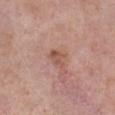{
  "biopsy_status": "not biopsied; imaged during a skin examination",
  "image": {
    "source": "total-body photography crop",
    "field_of_view_mm": 15
  },
  "lighting": "white-light",
  "site": "right lower leg",
  "lesion_size": {
    "long_diameter_mm_approx": 2.5
  },
  "automated_metrics": {
    "vs_skin_darker_L": 9.0,
    "vs_skin_contrast_norm": 6.5
  },
  "patient": {
    "sex": "male",
    "age_approx": 75
  }
}